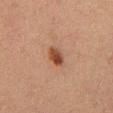• workup — catalogued during a skin exam; not biopsied
• site — the chest
• acquisition — ~15 mm crop, total-body skin-cancer survey
• subject — male, aged around 50